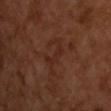follow-up=total-body-photography surveillance lesion; no biopsy
tile lighting=cross-polarized illumination
lesion size=about 4 mm
subject=male, roughly 65 years of age
image source=total-body-photography crop, ~15 mm field of view